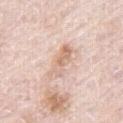Q: Is there a histopathology result?
A: total-body-photography surveillance lesion; no biopsy
Q: Illumination type?
A: white-light illumination
Q: Patient demographics?
A: male, approximately 80 years of age
Q: What kind of image is this?
A: 15 mm crop, total-body photography
Q: Lesion location?
A: the right thigh